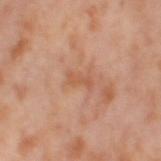Automated tile analysis of the lesion measured an eccentricity of roughly 0.9. The software also gave border irregularity of about 5 on a 0–10 scale, a color-variation rating of about 0/10, and radial color variation of about 0. And it measured a classifier nevus-likeness of about 0/100.
A female subject aged approximately 55.
A region of skin cropped from a whole-body photographic capture, roughly 15 mm wide.
On the left thigh.
About 2.5 mm across.
This is a cross-polarized tile.